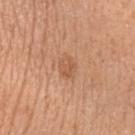workup: catalogued during a skin exam; not biopsied | size: about 2.5 mm | location: the left upper arm | patient: female, about 40 years old | illumination: white-light | imaging modality: ~15 mm tile from a whole-body skin photo.